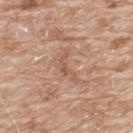This lesion was catalogued during total-body skin photography and was not selected for biopsy.
A close-up tile cropped from a whole-body skin photograph, about 15 mm across.
A male patient aged around 80.
On the upper back.